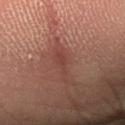Imaged during a routine full-body skin examination; the lesion was not biopsied and no histopathology is available.